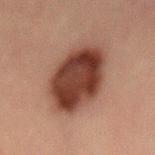workup = no biopsy performed (imaged during a skin exam)
patient = male, roughly 50 years of age
anatomic site = the lower back
imaging modality = total-body-photography crop, ~15 mm field of view
illumination = cross-polarized
diameter = ~7.5 mm (longest diameter)
image-analysis metrics = a footprint of about 31 mm² and a shape eccentricity near 0.75; a border-irregularity rating of about 1.5/10 and a within-lesion color-variation index near 5.5/10